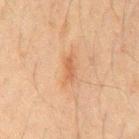{
  "biopsy_status": "not biopsied; imaged during a skin examination",
  "lighting": "cross-polarized",
  "image": {
    "source": "total-body photography crop",
    "field_of_view_mm": 15
  },
  "site": "chest",
  "lesion_size": {
    "long_diameter_mm_approx": 3.0
  },
  "patient": {
    "sex": "male",
    "age_approx": 60
  }
}